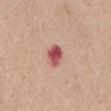biopsy status = total-body-photography surveillance lesion; no biopsy | tile lighting = white-light | imaging modality = ~15 mm crop, total-body skin-cancer survey | diameter = about 3 mm | location = the abdomen | patient = female, aged 63–67.